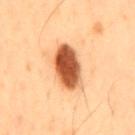<tbp_lesion>
<biopsy_status>not biopsied; imaged during a skin examination</biopsy_status>
<site>mid back</site>
<image>
  <source>total-body photography crop</source>
  <field_of_view_mm>15</field_of_view_mm>
</image>
<automated_metrics>
  <cielab_L>46</cielab_L>
  <cielab_a>24</cielab_a>
  <cielab_b>35</cielab_b>
  <vs_skin_contrast_norm>13.5</vs_skin_contrast_norm>
  <border_irregularity_0_10>1.5</border_irregularity_0_10>
  <peripheral_color_asymmetry>1.5</peripheral_color_asymmetry>
</automated_metrics>
<lesion_size>
  <long_diameter_mm_approx>5.5</long_diameter_mm_approx>
</lesion_size>
<patient>
  <sex>male</sex>
  <age_approx>55</age_approx>
</patient>
</tbp_lesion>